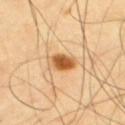TBP lesion metrics — a footprint of about 6.5 mm² and a shape-asymmetry score of about 0.2 (0 = symmetric); a classifier nevus-likeness of about 100/100 and lesion-presence confidence of about 100/100
acquisition — 15 mm crop, total-body photography
illumination — cross-polarized illumination
patient — male, in their mid- to late 60s
body site — the upper back
lesion size — about 3.5 mm